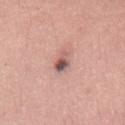workup — total-body-photography surveillance lesion; no biopsy
automated lesion analysis — a lesion area of about 4 mm² and a symmetry-axis asymmetry near 0.25; about 14 CIELAB-L* units darker than the surrounding skin and a normalized lesion–skin contrast near 9.5; a nevus-likeness score of about 80/100 and lesion-presence confidence of about 100/100
subject — female, roughly 25 years of age
diameter — about 3 mm
acquisition — 15 mm crop, total-body photography
body site — the abdomen
lighting — white-light illumination